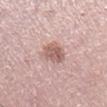<case>
<biopsy_status>not biopsied; imaged during a skin examination</biopsy_status>
<lesion_size>
  <long_diameter_mm_approx>3.5</long_diameter_mm_approx>
</lesion_size>
<site>right lower leg</site>
<lighting>white-light</lighting>
<automated_metrics>
  <area_mm2_approx>7.5</area_mm2_approx>
  <eccentricity>0.7</eccentricity>
  <shape_asymmetry>0.2</shape_asymmetry>
  <cielab_L>60</cielab_L>
  <cielab_a>20</cielab_a>
  <cielab_b>23</cielab_b>
  <vs_skin_darker_L>11.0</vs_skin_darker_L>
  <color_variation_0_10>3.0</color_variation_0_10>
  <peripheral_color_asymmetry>1.0</peripheral_color_asymmetry>
  <lesion_detection_confidence_0_100>100</lesion_detection_confidence_0_100>
</automated_metrics>
<image>
  <source>total-body photography crop</source>
  <field_of_view_mm>15</field_of_view_mm>
</image>
<patient>
  <sex>female</sex>
  <age_approx>40</age_approx>
</patient>
</case>